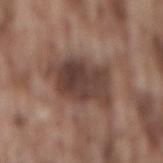The lesion was tiled from a total-body skin photograph and was not biopsied. The lesion's longest dimension is about 6.5 mm. The lesion is on the lower back. Automated tile analysis of the lesion measured a footprint of about 23 mm², an eccentricity of roughly 0.7, and a shape-asymmetry score of about 0.25 (0 = symmetric). It also reported a mean CIELAB color near L≈41 a*≈17 b*≈22, about 12 CIELAB-L* units darker than the surrounding skin, and a normalized border contrast of about 10. The analysis additionally found border irregularity of about 3 on a 0–10 scale and a within-lesion color-variation index near 5/10. It also reported a classifier nevus-likeness of about 10/100 and lesion-presence confidence of about 100/100. Captured under white-light illumination. A male patient aged around 75. A roughly 15 mm field-of-view crop from a total-body skin photograph.